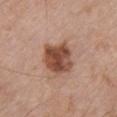Part of a total-body skin-imaging series; this lesion was reviewed on a skin check and was not flagged for biopsy. A 15 mm crop from a total-body photograph taken for skin-cancer surveillance. The lesion-visualizer software estimated a lesion area of about 13 mm², an outline eccentricity of about 0.45 (0 = round, 1 = elongated), and two-axis asymmetry of about 0.2. It also reported an automated nevus-likeness rating near 90 out of 100 and a lesion-detection confidence of about 100/100. A male subject, about 55 years old. The lesion is located on the chest. Imaged with white-light lighting.